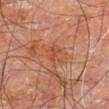<tbp_lesion>
  <lighting>cross-polarized</lighting>
  <image>
    <source>total-body photography crop</source>
    <field_of_view_mm>15</field_of_view_mm>
  </image>
  <patient>
    <sex>male</sex>
    <age_approx>60</age_approx>
  </patient>
  <lesion_size>
    <long_diameter_mm_approx>3.0</long_diameter_mm_approx>
  </lesion_size>
  <site>right leg</site>
</tbp_lesion>